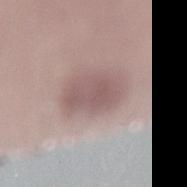anatomic site = the right lower leg; subject = female, about 40 years old; lesion size = ≈4.5 mm; image source = 15 mm crop, total-body photography.